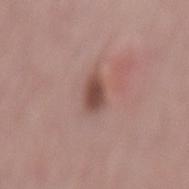biopsy_status: not biopsied; imaged during a skin examination
lighting: white-light
lesion_size:
  long_diameter_mm_approx: 3.0
site: back
image:
  source: total-body photography crop
  field_of_view_mm: 15
patient:
  sex: female
  age_approx: 65